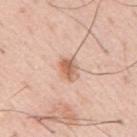TBP lesion metrics = a lesion–skin lightness drop of about 12; a nevus-likeness score of about 90/100 and a detector confidence of about 100 out of 100 that the crop contains a lesion | image = total-body-photography crop, ~15 mm field of view | size = ≈3 mm | tile lighting = white-light | patient = male, aged around 55 | body site = the mid back.